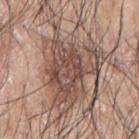The lesion was tiled from a total-body skin photograph and was not biopsied.
A male patient approximately 70 years of age.
Imaged with white-light lighting.
Located on the left arm.
The recorded lesion diameter is about 10.5 mm.
A roughly 15 mm field-of-view crop from a total-body skin photograph.